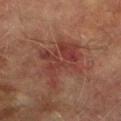Q: Was this lesion biopsied?
A: imaged on a skin check; not biopsied
Q: Lesion location?
A: the leg
Q: What kind of image is this?
A: total-body-photography crop, ~15 mm field of view
Q: Patient demographics?
A: male, aged 73 to 77
Q: What is the lesion's diameter?
A: ≈6.5 mm
Q: How was the tile lit?
A: cross-polarized illumination